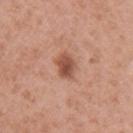Q: Who is the patient?
A: female, aged approximately 30
Q: How was the tile lit?
A: white-light illumination
Q: What is the anatomic site?
A: the arm
Q: How was this image acquired?
A: ~15 mm tile from a whole-body skin photo
Q: Lesion size?
A: ≈3.5 mm